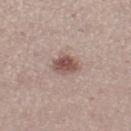Case summary:
– workup · catalogued during a skin exam; not biopsied
– imaging modality · ~15 mm crop, total-body skin-cancer survey
– anatomic site · the left thigh
– TBP lesion metrics · a border-irregularity rating of about 2/10, a within-lesion color-variation index near 4/10, and peripheral color asymmetry of about 1; an automated nevus-likeness rating near 85 out of 100 and a detector confidence of about 100 out of 100 that the crop contains a lesion
– lesion size · ~3 mm (longest diameter)
– patient · female, aged 18 to 22
– lighting · white-light